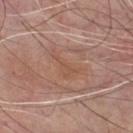No biopsy was performed on this lesion — it was imaged during a full skin examination and was not determined to be concerning.
From the chest.
The patient is a male in their mid-70s.
A lesion tile, about 15 mm wide, cut from a 3D total-body photograph.